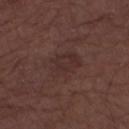{
  "biopsy_status": "not biopsied; imaged during a skin examination",
  "automated_metrics": {
    "area_mm2_approx": 10.0,
    "eccentricity": 0.7,
    "shape_asymmetry": 0.2,
    "cielab_L": 29,
    "cielab_a": 17,
    "cielab_b": 18,
    "vs_skin_darker_L": 4.0,
    "vs_skin_contrast_norm": 5.0,
    "peripheral_color_asymmetry": 1.0
  },
  "lesion_size": {
    "long_diameter_mm_approx": 4.0
  },
  "lighting": "white-light",
  "patient": {
    "sex": "male",
    "age_approx": 75
  },
  "image": {
    "source": "total-body photography crop",
    "field_of_view_mm": 15
  },
  "site": "right forearm"
}